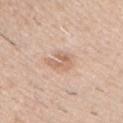notes — imaged on a skin check; not biopsied | imaging modality — total-body-photography crop, ~15 mm field of view | image-analysis metrics — a mean CIELAB color near L≈64 a*≈19 b*≈30, about 9 CIELAB-L* units darker than the surrounding skin, and a normalized border contrast of about 6; a classifier nevus-likeness of about 5/100 and a detector confidence of about 100 out of 100 that the crop contains a lesion | anatomic site — the chest | tile lighting — white-light | subject — male, aged 38 to 42.